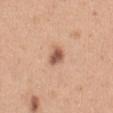  biopsy_status: not biopsied; imaged during a skin examination
  image:
    source: total-body photography crop
    field_of_view_mm: 15
  patient:
    sex: female
    age_approx: 30
  lesion_size:
    long_diameter_mm_approx: 2.5
  site: abdomen